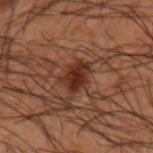Clinical impression:
No biopsy was performed on this lesion — it was imaged during a full skin examination and was not determined to be concerning.
Background:
The patient is a male in their 50s. On the right forearm. A 15 mm crop from a total-body photograph taken for skin-cancer surveillance. Captured under cross-polarized illumination. Longest diameter approximately 3 mm.